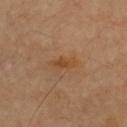Clinical impression: Captured during whole-body skin photography for melanoma surveillance; the lesion was not biopsied. Background: The lesion-visualizer software estimated a lesion color around L≈47 a*≈21 b*≈37 in CIELAB and a normalized border contrast of about 7. The software also gave a border-irregularity index near 3.5/10 and a color-variation rating of about 1/10. The lesion's longest dimension is about 3.5 mm. Imaged with cross-polarized lighting. A region of skin cropped from a whole-body photographic capture, roughly 15 mm wide. The lesion is on the chest.